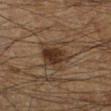biopsy_status: not biopsied; imaged during a skin examination
patient:
  sex: male
  age_approx: 65
lesion_size:
  long_diameter_mm_approx: 4.0
automated_metrics:
  border_irregularity_0_10: 4.0
  peripheral_color_asymmetry: 1.5
  nevus_likeness_0_100: 90
  lesion_detection_confidence_0_100: 100
image:
  source: total-body photography crop
  field_of_view_mm: 15
lighting: cross-polarized
site: left lower leg